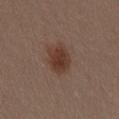notes = no biopsy performed (imaged during a skin exam); body site = the back; lighting = white-light illumination; lesion size = ~4 mm (longest diameter); patient = female, in their 30s; acquisition = total-body-photography crop, ~15 mm field of view.